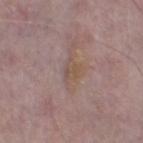Q: Was this lesion biopsied?
A: catalogued during a skin exam; not biopsied
Q: What is the anatomic site?
A: the left thigh
Q: What are the patient's age and sex?
A: male, in their mid- to late 60s
Q: How was this image acquired?
A: total-body-photography crop, ~15 mm field of view